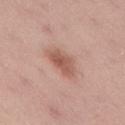Impression:
Part of a total-body skin-imaging series; this lesion was reviewed on a skin check and was not flagged for biopsy.
Clinical summary:
The lesion's longest dimension is about 4 mm. Captured under white-light illumination. A roughly 15 mm field-of-view crop from a total-body skin photograph. Automated image analysis of the tile measured an average lesion color of about L≈56 a*≈23 b*≈27 (CIELAB), a lesion–skin lightness drop of about 11, and a normalized border contrast of about 7.5. The software also gave a nevus-likeness score of about 90/100 and a lesion-detection confidence of about 100/100. A male patient, about 55 years old. The lesion is on the lower back.